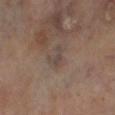From the left lower leg.
Cropped from a total-body skin-imaging series; the visible field is about 15 mm.
The subject is a male about 70 years old.